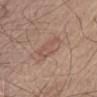Case summary:
– notes · imaged on a skin check; not biopsied
– size · about 5 mm
– illumination · white-light illumination
– site · the front of the torso
– image source · total-body-photography crop, ~15 mm field of view
– patient · male, aged 68–72